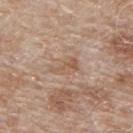The lesion was photographed on a routine skin check and not biopsied; there is no pathology result.
The lesion-visualizer software estimated roughly 7 lightness units darker than nearby skin and a lesion-to-skin contrast of about 5.5 (normalized; higher = more distinct). The analysis additionally found a border-irregularity rating of about 4.5/10, a within-lesion color-variation index near 3.5/10, and peripheral color asymmetry of about 1. And it measured a classifier nevus-likeness of about 0/100 and a detector confidence of about 100 out of 100 that the crop contains a lesion.
A male patient, in their 80s.
A lesion tile, about 15 mm wide, cut from a 3D total-body photograph.
Located on the upper back.
Approximately 3.5 mm at its widest.
This is a white-light tile.